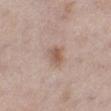No biopsy was performed on this lesion — it was imaged during a full skin examination and was not determined to be concerning.
The lesion-visualizer software estimated a mean CIELAB color near L≈57 a*≈17 b*≈26, roughly 9 lightness units darker than nearby skin, and a normalized lesion–skin contrast near 7. The analysis additionally found a within-lesion color-variation index near 4.5/10 and radial color variation of about 1.5. And it measured an automated nevus-likeness rating near 60 out of 100 and a lesion-detection confidence of about 100/100.
A roughly 15 mm field-of-view crop from a total-body skin photograph.
Measured at roughly 2.5 mm in maximum diameter.
Located on the left lower leg.
The patient is a female about 40 years old.